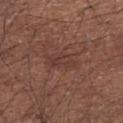Assessment: Recorded during total-body skin imaging; not selected for excision or biopsy. Context: Located on the left upper arm. A 15 mm close-up tile from a total-body photography series done for melanoma screening. A male subject in their mid- to late 60s. Automated tile analysis of the lesion measured a lesion color around L≈37 a*≈20 b*≈23 in CIELAB, a lesion–skin lightness drop of about 5, and a lesion-to-skin contrast of about 5 (normalized; higher = more distinct). The analysis additionally found a border-irregularity index near 4/10 and peripheral color asymmetry of about 0.5. The software also gave a classifier nevus-likeness of about 5/100. Approximately 3.5 mm at its widest. Imaged with white-light lighting.